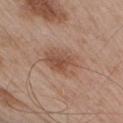Imaged during a routine full-body skin examination; the lesion was not biopsied and no histopathology is available.
The lesion is on the arm.
About 4 mm across.
Cropped from a whole-body photographic skin survey; the tile spans about 15 mm.
The tile uses white-light illumination.
The patient is a male roughly 55 years of age.